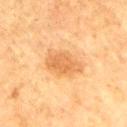Q: Is there a histopathology result?
A: no biopsy performed (imaged during a skin exam)
Q: Who is the patient?
A: male, aged 63–67
Q: What kind of image is this?
A: ~15 mm crop, total-body skin-cancer survey
Q: What lighting was used for the tile?
A: cross-polarized illumination
Q: How large is the lesion?
A: ≈5 mm
Q: What is the anatomic site?
A: the arm